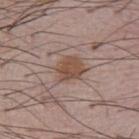Impression:
Imaged during a routine full-body skin examination; the lesion was not biopsied and no histopathology is available.
Clinical summary:
This is a white-light tile. A 15 mm close-up extracted from a 3D total-body photography capture. An algorithmic analysis of the crop reported a lesion color around L≈49 a*≈18 b*≈26 in CIELAB, roughly 9 lightness units darker than nearby skin, and a normalized border contrast of about 8. It also reported a within-lesion color-variation index near 3/10 and a peripheral color-asymmetry measure near 1. It also reported a nevus-likeness score of about 90/100 and a detector confidence of about 100 out of 100 that the crop contains a lesion. Measured at roughly 3.5 mm in maximum diameter. A male patient, approximately 55 years of age. The lesion is located on the chest.